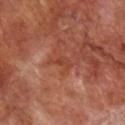workup: catalogued during a skin exam; not biopsied
image: total-body-photography crop, ~15 mm field of view
subject: male, aged 68–72
anatomic site: the right lower leg